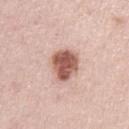Recorded during total-body skin imaging; not selected for excision or biopsy. Located on the left upper arm. A 15 mm close-up extracted from a 3D total-body photography capture. Measured at roughly 4 mm in maximum diameter. Imaged with white-light lighting. A male patient, aged 43 to 47.